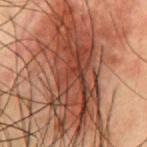Impression: Imaged during a routine full-body skin examination; the lesion was not biopsied and no histopathology is available. Image and clinical context: Captured under cross-polarized illumination. Approximately 14 mm at its widest. From the front of the torso. The total-body-photography lesion software estimated a lesion area of about 60 mm², an eccentricity of roughly 0.9, and two-axis asymmetry of about 0.35. And it measured a mean CIELAB color near L≈36 a*≈22 b*≈26 and a normalized border contrast of about 11. The analysis additionally found a nevus-likeness score of about 20/100. The subject is a male roughly 55 years of age. Cropped from a whole-body photographic skin survey; the tile spans about 15 mm.